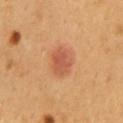follow-up: imaged on a skin check; not biopsied.